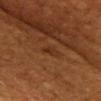Assessment:
No biopsy was performed on this lesion — it was imaged during a full skin examination and was not determined to be concerning.
Acquisition and patient details:
A region of skin cropped from a whole-body photographic capture, roughly 15 mm wide. This is a cross-polarized tile. The total-body-photography lesion software estimated an area of roughly 4 mm² and an outline eccentricity of about 0.8 (0 = round, 1 = elongated). And it measured a mean CIELAB color near L≈25 a*≈19 b*≈27 and about 5 CIELAB-L* units darker than the surrounding skin. A female patient roughly 50 years of age. From the head or neck.